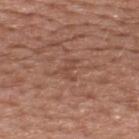Clinical impression:
Recorded during total-body skin imaging; not selected for excision or biopsy.
Context:
The lesion is on the upper back. Imaged with white-light lighting. A male patient, roughly 55 years of age. An algorithmic analysis of the crop reported an area of roughly 3 mm², an eccentricity of roughly 0.75, and a symmetry-axis asymmetry near 0.6. The recorded lesion diameter is about 2.5 mm. This image is a 15 mm lesion crop taken from a total-body photograph.